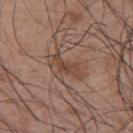biopsy status = total-body-photography surveillance lesion; no biopsy
imaging modality = ~15 mm crop, total-body skin-cancer survey
body site = the upper back
lesion diameter = ≈3.5 mm
subject = male, roughly 50 years of age
lighting = white-light illumination